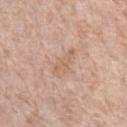  image:
    source: total-body photography crop
    field_of_view_mm: 15
  lesion_size:
    long_diameter_mm_approx: 4.0
  site: left forearm
  automated_metrics:
    cielab_L: 63
    cielab_a: 18
    cielab_b: 31
  lighting: white-light
  patient:
    sex: female
    age_approx: 65